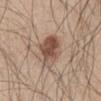A close-up tile cropped from a whole-body skin photograph, about 15 mm across. The lesion is located on the left thigh. The tile uses white-light illumination. A male patient aged 58 to 62. Automated tile analysis of the lesion measured an outline eccentricity of about 0.85 (0 = round, 1 = elongated) and a shape-asymmetry score of about 0.2 (0 = symmetric). It also reported a classifier nevus-likeness of about 95/100 and a lesion-detection confidence of about 100/100.